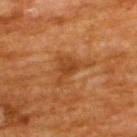Case summary:
- biopsy status: no biopsy performed (imaged during a skin exam)
- lesion diameter: about 5 mm
- image: ~15 mm crop, total-body skin-cancer survey
- illumination: cross-polarized illumination
- patient: male, aged approximately 60
- body site: the upper back